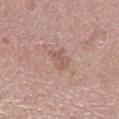notes — catalogued during a skin exam; not biopsied | image — ~15 mm tile from a whole-body skin photo | anatomic site — the left lower leg | size — ≈3 mm | automated metrics — a lesion area of about 5 mm², a shape eccentricity near 0.6, and two-axis asymmetry of about 0.3; border irregularity of about 3 on a 0–10 scale, internal color variation of about 2.5 on a 0–10 scale, and a peripheral color-asymmetry measure near 1; a classifier nevus-likeness of about 0/100 and a lesion-detection confidence of about 100/100 | subject — male, aged 63 to 67.